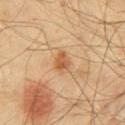The lesion was photographed on a routine skin check and not biopsied; there is no pathology result.
A close-up tile cropped from a whole-body skin photograph, about 15 mm across.
This is a cross-polarized tile.
A male subject, aged 38–42.
Located on the mid back.
The lesion-visualizer software estimated a lesion area of about 4 mm² and a shape-asymmetry score of about 0.25 (0 = symmetric). The analysis additionally found a lesion-detection confidence of about 100/100.